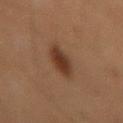– biopsy status: no biopsy performed (imaged during a skin exam)
– tile lighting: cross-polarized
– lesion size: ~4 mm (longest diameter)
– image source: 15 mm crop, total-body photography
– subject: male, aged approximately 60
– automated lesion analysis: a footprint of about 8 mm², an eccentricity of roughly 0.8, and a symmetry-axis asymmetry near 0.1; a lesion–skin lightness drop of about 10 and a lesion-to-skin contrast of about 9 (normalized; higher = more distinct); a classifier nevus-likeness of about 100/100 and a detector confidence of about 100 out of 100 that the crop contains a lesion
– anatomic site: the abdomen